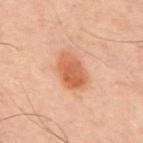Q: Is there a histopathology result?
A: catalogued during a skin exam; not biopsied
Q: What did automated image analysis measure?
A: a border-irregularity rating of about 1.5/10, a within-lesion color-variation index near 4.5/10, and radial color variation of about 1.5
Q: What is the imaging modality?
A: ~15 mm tile from a whole-body skin photo
Q: Lesion location?
A: the back
Q: How was the tile lit?
A: cross-polarized illumination
Q: Who is the patient?
A: male, aged 48–52